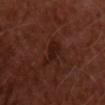Q: Where on the body is the lesion?
A: the front of the torso
Q: Lesion size?
A: about 4 mm
Q: What kind of image is this?
A: ~15 mm crop, total-body skin-cancer survey
Q: Patient demographics?
A: male, in their 50s
Q: What lighting was used for the tile?
A: cross-polarized
Q: What did automated image analysis measure?
A: a classifier nevus-likeness of about 5/100